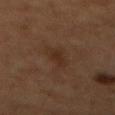Case summary:
- biopsy status — catalogued during a skin exam; not biopsied
- body site — the back
- illumination — cross-polarized illumination
- size — ≈4 mm
- patient — male, roughly 60 years of age
- image source — 15 mm crop, total-body photography